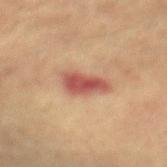The lesion was tiled from a total-body skin photograph and was not biopsied. A female patient approximately 80 years of age. A 15 mm close-up tile from a total-body photography series done for melanoma screening. Imaged with cross-polarized lighting. Longest diameter approximately 4.5 mm. From the mid back.